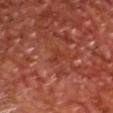Case summary:
* workup · imaged on a skin check; not biopsied
* image source · ~15 mm crop, total-body skin-cancer survey
* illumination · cross-polarized
* patient · male, aged approximately 65
* diameter · ~2.5 mm (longest diameter)